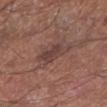<record>
<automated_metrics>
  <color_variation_0_10>2.5</color_variation_0_10>
  <peripheral_color_asymmetry>1.0</peripheral_color_asymmetry>
  <nevus_likeness_0_100>0</nevus_likeness_0_100>
  <lesion_detection_confidence_0_100>100</lesion_detection_confidence_0_100>
</automated_metrics>
<site>left lower leg</site>
<patient>
  <sex>male</sex>
  <age_approx>70</age_approx>
</patient>
<lighting>white-light</lighting>
<image>
  <source>total-body photography crop</source>
  <field_of_view_mm>15</field_of_view_mm>
</image>
</record>